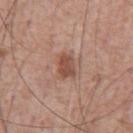– subject · male, aged around 60
– lighting · white-light
– size · ≈3 mm
– TBP lesion metrics · a shape eccentricity near 0.7 and two-axis asymmetry of about 0.25; a mean CIELAB color near L≈50 a*≈21 b*≈28, roughly 10 lightness units darker than nearby skin, and a lesion-to-skin contrast of about 7.5 (normalized; higher = more distinct)
– imaging modality · ~15 mm crop, total-body skin-cancer survey
– body site · the chest